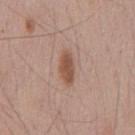Part of a total-body skin-imaging series; this lesion was reviewed on a skin check and was not flagged for biopsy. A male patient, aged 63–67. Measured at roughly 4 mm in maximum diameter. The lesion is located on the chest. This is a white-light tile. A close-up tile cropped from a whole-body skin photograph, about 15 mm across. The total-body-photography lesion software estimated an area of roughly 6.5 mm², an eccentricity of roughly 0.9, and a shape-asymmetry score of about 0.35 (0 = symmetric). It also reported an average lesion color of about L≈52 a*≈20 b*≈28 (CIELAB), a lesion–skin lightness drop of about 11, and a normalized border contrast of about 8.5.